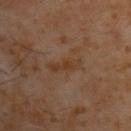Impression:
Part of a total-body skin-imaging series; this lesion was reviewed on a skin check and was not flagged for biopsy.
Acquisition and patient details:
Longest diameter approximately 4.5 mm. A male patient, roughly 60 years of age. Cropped from a whole-body photographic skin survey; the tile spans about 15 mm. From the back. The tile uses cross-polarized illumination.